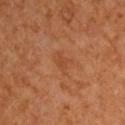The lesion was tiled from a total-body skin photograph and was not biopsied. The recorded lesion diameter is about 3 mm. From the right upper arm. Automated tile analysis of the lesion measured a footprint of about 4.5 mm² and an eccentricity of roughly 0.75. Cropped from a total-body skin-imaging series; the visible field is about 15 mm. A male subject aged 63–67.